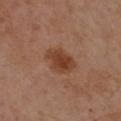Impression:
No biopsy was performed on this lesion — it was imaged during a full skin examination and was not determined to be concerning.
Background:
This is a cross-polarized tile. From the left forearm. The subject is a female in their 40s. Automated tile analysis of the lesion measured a footprint of about 8.5 mm², an eccentricity of roughly 0.7, and a shape-asymmetry score of about 0.25 (0 = symmetric). It also reported an average lesion color of about L≈41 a*≈22 b*≈31 (CIELAB) and a lesion–skin lightness drop of about 10. And it measured border irregularity of about 2 on a 0–10 scale, internal color variation of about 3 on a 0–10 scale, and radial color variation of about 1. A 15 mm close-up tile from a total-body photography series done for melanoma screening.